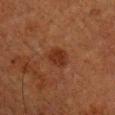acquisition=15 mm crop, total-body photography | lesion diameter=~2.5 mm (longest diameter) | body site=the right upper arm | patient=male, approximately 75 years of age | image-analysis metrics=a lesion area of about 5 mm², an eccentricity of roughly 0.5, and a symmetry-axis asymmetry near 0.2; a lesion color around L≈26 a*≈21 b*≈27 in CIELAB, about 7 CIELAB-L* units darker than the surrounding skin, and a normalized border contrast of about 7.5; a nevus-likeness score of about 85/100 and a lesion-detection confidence of about 100/100 | illumination=cross-polarized illumination.